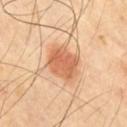<lesion>
<biopsy_status>not biopsied; imaged during a skin examination</biopsy_status>
<image>
  <source>total-body photography crop</source>
  <field_of_view_mm>15</field_of_view_mm>
</image>
<site>chest</site>
<lesion_size>
  <long_diameter_mm_approx>4.5</long_diameter_mm_approx>
</lesion_size>
<lighting>cross-polarized</lighting>
</lesion>